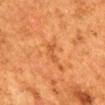Clinical impression:
This lesion was catalogued during total-body skin photography and was not selected for biopsy.
Context:
Captured under cross-polarized illumination. The lesion is on the mid back. A female subject approximately 50 years of age. Measured at roughly 2.5 mm in maximum diameter. A region of skin cropped from a whole-body photographic capture, roughly 15 mm wide.